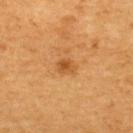Q: Was a biopsy performed?
A: no biopsy performed (imaged during a skin exam)
Q: How large is the lesion?
A: about 2.5 mm
Q: Where on the body is the lesion?
A: the upper back
Q: What kind of image is this?
A: total-body-photography crop, ~15 mm field of view
Q: What are the patient's age and sex?
A: male, in their 50s
Q: What lighting was used for the tile?
A: cross-polarized illumination